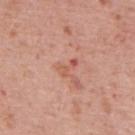No biopsy was performed on this lesion — it was imaged during a full skin examination and was not determined to be concerning.
A male subject aged approximately 70.
The lesion is on the mid back.
Cropped from a whole-body photographic skin survey; the tile spans about 15 mm.
The tile uses white-light illumination.
The lesion's longest dimension is about 2.5 mm.